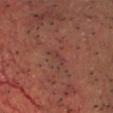Q: Is there a histopathology result?
A: catalogued during a skin exam; not biopsied
Q: What is the lesion's diameter?
A: ~2.5 mm (longest diameter)
Q: What kind of image is this?
A: ~15 mm tile from a whole-body skin photo
Q: What lighting was used for the tile?
A: cross-polarized
Q: What is the anatomic site?
A: the chest
Q: Patient demographics?
A: male, aged 63 to 67
Q: Automated lesion metrics?
A: a lesion area of about 2.5 mm², an eccentricity of roughly 0.95, and a symmetry-axis asymmetry near 0.45; a mean CIELAB color near L≈37 a*≈24 b*≈22, a lesion–skin lightness drop of about 5, and a normalized border contrast of about 4.5; lesion-presence confidence of about 85/100